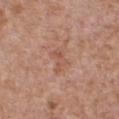Q: What lighting was used for the tile?
A: white-light illumination
Q: What are the patient's age and sex?
A: male, aged 63 to 67
Q: Lesion location?
A: the chest
Q: Automated lesion metrics?
A: a lesion area of about 3.5 mm², a shape eccentricity near 0.85, and a shape-asymmetry score of about 0.55 (0 = symmetric); a lesion-detection confidence of about 100/100
Q: How large is the lesion?
A: about 3 mm
Q: How was this image acquired?
A: total-body-photography crop, ~15 mm field of view